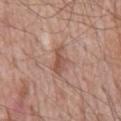The lesion was photographed on a routine skin check and not biopsied; there is no pathology result.
This image is a 15 mm lesion crop taken from a total-body photograph.
About 3.5 mm across.
From the mid back.
A male patient, in their 60s.
The tile uses white-light illumination.
Automated tile analysis of the lesion measured an area of roughly 5 mm², an eccentricity of roughly 0.85, and a symmetry-axis asymmetry near 0.4. And it measured a lesion color around L≈53 a*≈21 b*≈27 in CIELAB and a lesion-to-skin contrast of about 7 (normalized; higher = more distinct). The software also gave border irregularity of about 4.5 on a 0–10 scale and a within-lesion color-variation index near 2.5/10. It also reported a lesion-detection confidence of about 100/100.